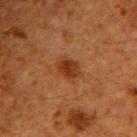Image and clinical context: This is a cross-polarized tile. A roughly 15 mm field-of-view crop from a total-body skin photograph. A male subject approximately 60 years of age. An algorithmic analysis of the crop reported a lesion area of about 5 mm², a shape eccentricity near 0.65, and two-axis asymmetry of about 0.25. It also reported a color-variation rating of about 3.5/10 and a peripheral color-asymmetry measure near 1. It also reported a classifier nevus-likeness of about 90/100 and a detector confidence of about 100 out of 100 that the crop contains a lesion. Located on the right upper arm.